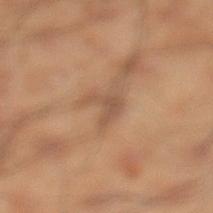follow-up=catalogued during a skin exam; not biopsied
image source=total-body-photography crop, ~15 mm field of view
size=about 3 mm
lighting=cross-polarized
anatomic site=the left lower leg
subject=male, about 50 years old